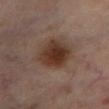Findings:
• patient — male, aged approximately 70
• automated metrics — a lesion area of about 17 mm², an outline eccentricity of about 0.45 (0 = round, 1 = elongated), and a symmetry-axis asymmetry near 0.15; an average lesion color of about L≈34 a*≈17 b*≈24 (CIELAB), about 10 CIELAB-L* units darker than the surrounding skin, and a normalized border contrast of about 10; border irregularity of about 2 on a 0–10 scale, a color-variation rating of about 5/10, and peripheral color asymmetry of about 1.5; lesion-presence confidence of about 100/100
• body site — the leg
• tile lighting — cross-polarized
• image — total-body-photography crop, ~15 mm field of view
• lesion size — about 4.5 mm